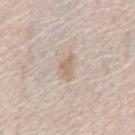{
  "patient": {
    "sex": "male",
    "age_approx": 75
  },
  "image": {
    "source": "total-body photography crop",
    "field_of_view_mm": 15
  },
  "lesion_size": {
    "long_diameter_mm_approx": 3.0
  },
  "lighting": "white-light",
  "site": "abdomen"
}